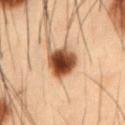{"biopsy_status": "not biopsied; imaged during a skin examination", "site": "abdomen", "image": {"source": "total-body photography crop", "field_of_view_mm": 15}, "lesion_size": {"long_diameter_mm_approx": 4.0}, "patient": {"sex": "male", "age_approx": 55}, "automated_metrics": {"cielab_L": 39, "cielab_a": 20, "cielab_b": 30, "vs_skin_darker_L": 20.0, "vs_skin_contrast_norm": 15.0}, "lighting": "cross-polarized"}